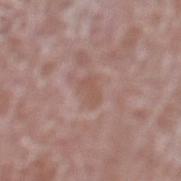Part of a total-body skin-imaging series; this lesion was reviewed on a skin check and was not flagged for biopsy.
The tile uses white-light illumination.
A male patient, aged 73–77.
From the leg.
A 15 mm close-up extracted from a 3D total-body photography capture.
Longest diameter approximately 2.5 mm.